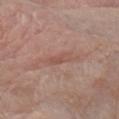workup = no biopsy performed (imaged during a skin exam) | imaging modality = ~15 mm crop, total-body skin-cancer survey | patient = female, in their mid-60s | location = the left forearm.